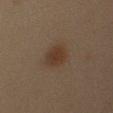Q: Was a biopsy performed?
A: imaged on a skin check; not biopsied
Q: How was this image acquired?
A: ~15 mm crop, total-body skin-cancer survey
Q: Where on the body is the lesion?
A: the arm
Q: Illumination type?
A: cross-polarized illumination
Q: What is the lesion's diameter?
A: ~3.5 mm (longest diameter)
Q: Who is the patient?
A: male, about 45 years old